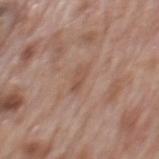Clinical impression: Part of a total-body skin-imaging series; this lesion was reviewed on a skin check and was not flagged for biopsy. Acquisition and patient details: A male patient, about 70 years old. From the mid back. Cropped from a whole-body photographic skin survey; the tile spans about 15 mm.